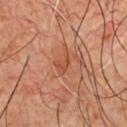Recorded during total-body skin imaging; not selected for excision or biopsy. A male subject aged around 60. About 3 mm across. On the chest. A roughly 15 mm field-of-view crop from a total-body skin photograph.